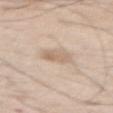biopsy_status: not biopsied; imaged during a skin examination
lighting: white-light
automated_metrics:
  area_mm2_approx: 6.5
  shape_asymmetry: 0.2
  vs_skin_darker_L: 9.0
  vs_skin_contrast_norm: 5.5
  lesion_detection_confidence_0_100: 100
site: abdomen
patient:
  sex: male
  age_approx: 70
lesion_size:
  long_diameter_mm_approx: 3.0
image:
  source: total-body photography crop
  field_of_view_mm: 15